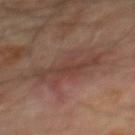{
  "biopsy_status": "not biopsied; imaged during a skin examination",
  "automated_metrics": {
    "cielab_L": 39,
    "cielab_a": 19,
    "cielab_b": 23,
    "vs_skin_darker_L": 6.0,
    "vs_skin_contrast_norm": 5.5,
    "border_irregularity_0_10": 5.0,
    "color_variation_0_10": 3.5,
    "peripheral_color_asymmetry": 1.0,
    "nevus_likeness_0_100": 5,
    "lesion_detection_confidence_0_100": 90
  },
  "site": "mid back",
  "lesion_size": {
    "long_diameter_mm_approx": 6.5
  },
  "image": {
    "source": "total-body photography crop",
    "field_of_view_mm": 15
  },
  "lighting": "cross-polarized",
  "patient": {
    "sex": "male",
    "age_approx": 65
  }
}